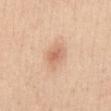notes: catalogued during a skin exam; not biopsied | anatomic site: the front of the torso | size: ≈3 mm | subject: female, in their 30s | image: ~15 mm crop, total-body skin-cancer survey.